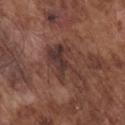Q: Is there a histopathology result?
A: total-body-photography surveillance lesion; no biopsy
Q: How large is the lesion?
A: ≈6 mm
Q: What is the anatomic site?
A: the chest
Q: What lighting was used for the tile?
A: white-light illumination
Q: Patient demographics?
A: male, aged around 75
Q: Automated lesion metrics?
A: a footprint of about 11 mm² and a shape-asymmetry score of about 0.5 (0 = symmetric); a mean CIELAB color near L≈35 a*≈19 b*≈21 and a lesion-to-skin contrast of about 8.5 (normalized; higher = more distinct); a border-irregularity index near 8.5/10; a classifier nevus-likeness of about 0/100
Q: How was this image acquired?
A: total-body-photography crop, ~15 mm field of view